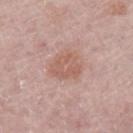The lesion is located on the left lower leg. Cropped from a whole-body photographic skin survey; the tile spans about 15 mm. The subject is a female aged 63–67.